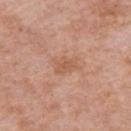  lesion_size:
    long_diameter_mm_approx: 2.5
  site: arm
  automated_metrics:
    area_mm2_approx: 4.0
    eccentricity: 0.65
    shape_asymmetry: 0.3
    cielab_L: 57
    cielab_a: 23
    cielab_b: 32
    vs_skin_darker_L: 7.0
    vs_skin_contrast_norm: 5.0
    lesion_detection_confidence_0_100: 100
  image:
    source: total-body photography crop
    field_of_view_mm: 15
  patient:
    sex: female
    age_approx: 70
  lighting: white-light